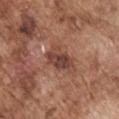biopsy status = no biopsy performed (imaged during a skin exam) | location = the arm | lesion size = about 4 mm | subject = male, about 75 years old | acquisition = ~15 mm tile from a whole-body skin photo | tile lighting = white-light illumination.